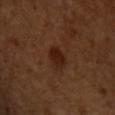On the chest.
Imaged with cross-polarized lighting.
A 15 mm close-up extracted from a 3D total-body photography capture.
The lesion's longest dimension is about 2.5 mm.
A male patient about 50 years old.